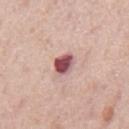| key | value |
|---|---|
| workup | no biopsy performed (imaged during a skin exam) |
| location | the back |
| image | total-body-photography crop, ~15 mm field of view |
| patient | male, in their mid- to late 70s |
| lighting | white-light |
| size | ~3 mm (longest diameter) |